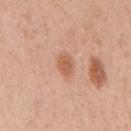{
  "biopsy_status": "not biopsied; imaged during a skin examination",
  "image": {
    "source": "total-body photography crop",
    "field_of_view_mm": 15
  },
  "patient": {
    "sex": "male",
    "age_approx": 55
  },
  "site": "chest",
  "lighting": "white-light"
}